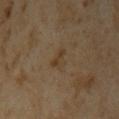A female subject, roughly 35 years of age. This is a cross-polarized tile. On the upper back. The lesion's longest dimension is about 3 mm. A lesion tile, about 15 mm wide, cut from a 3D total-body photograph.